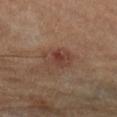Clinical impression:
Part of a total-body skin-imaging series; this lesion was reviewed on a skin check and was not flagged for biopsy.
Context:
A 15 mm close-up extracted from a 3D total-body photography capture. On the leg. The patient is a male aged 83 or over.